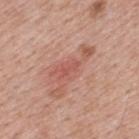A region of skin cropped from a whole-body photographic capture, roughly 15 mm wide. Measured at roughly 2.5 mm in maximum diameter. The tile uses white-light illumination. Located on the upper back. The patient is a male approximately 40 years of age.